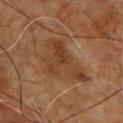Captured during whole-body skin photography for melanoma surveillance; the lesion was not biopsied.